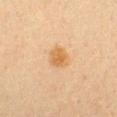• biopsy status · total-body-photography surveillance lesion; no biopsy
• location · the chest
• subject · female, approximately 20 years of age
• lighting · cross-polarized illumination
• image · ~15 mm crop, total-body skin-cancer survey